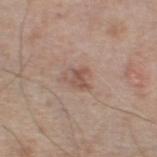<case>
<patient>
  <sex>male</sex>
  <age_approx>65</age_approx>
</patient>
<image>
  <source>total-body photography crop</source>
  <field_of_view_mm>15</field_of_view_mm>
</image>
<lesion_size>
  <long_diameter_mm_approx>2.5</long_diameter_mm_approx>
</lesion_size>
<lighting>white-light</lighting>
<site>left thigh</site>
</case>